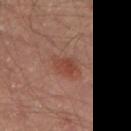Recorded during total-body skin imaging; not selected for excision or biopsy.
A 15 mm crop from a total-body photograph taken for skin-cancer surveillance.
A male patient, aged approximately 65.
The lesion is located on the left thigh.